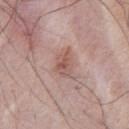This lesion was catalogued during total-body skin photography and was not selected for biopsy.
A region of skin cropped from a whole-body photographic capture, roughly 15 mm wide.
The tile uses white-light illumination.
The lesion is located on the chest.
The lesion's longest dimension is about 3.5 mm.
A male patient, approximately 40 years of age.
The total-body-photography lesion software estimated an outline eccentricity of about 0.7 (0 = round, 1 = elongated). It also reported a lesion color around L≈56 a*≈21 b*≈23 in CIELAB, a lesion–skin lightness drop of about 9, and a lesion-to-skin contrast of about 6 (normalized; higher = more distinct). It also reported a classifier nevus-likeness of about 50/100 and a lesion-detection confidence of about 100/100.